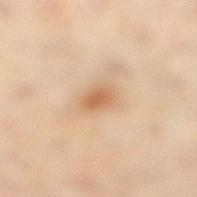Part of a total-body skin-imaging series; this lesion was reviewed on a skin check and was not flagged for biopsy.
A 15 mm close-up tile from a total-body photography series done for melanoma screening.
From the right lower leg.
Automated image analysis of the tile measured a mean CIELAB color near L≈65 a*≈19 b*≈37, a lesion–skin lightness drop of about 10, and a normalized lesion–skin contrast near 7. The software also gave a border-irregularity rating of about 1.5/10 and internal color variation of about 3 on a 0–10 scale. The analysis additionally found a classifier nevus-likeness of about 60/100 and a lesion-detection confidence of about 100/100.
This is a cross-polarized tile.
A female patient aged 43–47.